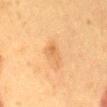notes: catalogued during a skin exam; not biopsied | subject: female, aged around 50 | lesion size: ~3 mm (longest diameter) | anatomic site: the lower back | automated lesion analysis: an average lesion color of about L≈55 a*≈20 b*≈38 (CIELAB), a lesion–skin lightness drop of about 7, and a normalized border contrast of about 5; an automated nevus-likeness rating near 25 out of 100 and lesion-presence confidence of about 100/100 | illumination: cross-polarized | image source: ~15 mm crop, total-body skin-cancer survey.